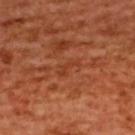| field | value |
|---|---|
| patient | female, in their mid-50s |
| anatomic site | the upper back |
| imaging modality | 15 mm crop, total-body photography |
| size | about 3 mm |
| illumination | cross-polarized illumination |